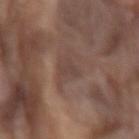Part of a total-body skin-imaging series; this lesion was reviewed on a skin check and was not flagged for biopsy.